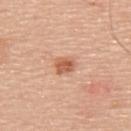{"biopsy_status": "not biopsied; imaged during a skin examination", "lesion_size": {"long_diameter_mm_approx": 2.5}, "site": "upper back", "patient": {"sex": "male", "age_approx": 70}, "image": {"source": "total-body photography crop", "field_of_view_mm": 15}, "automated_metrics": {"area_mm2_approx": 4.5, "eccentricity": 0.65, "cielab_L": 59, "cielab_a": 25, "cielab_b": 34, "vs_skin_darker_L": 12.0, "vs_skin_contrast_norm": 8.0, "border_irregularity_0_10": 1.5, "nevus_likeness_0_100": 90, "lesion_detection_confidence_0_100": 100}}